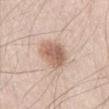Recorded during total-body skin imaging; not selected for excision or biopsy. The lesion is located on the right thigh. Longest diameter approximately 3.5 mm. Imaged with white-light lighting. A male subject, aged 38–42. This image is a 15 mm lesion crop taken from a total-body photograph.